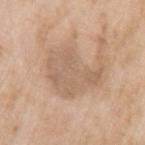workup: no biopsy performed (imaged during a skin exam)
automated lesion analysis: an average lesion color of about L≈61 a*≈16 b*≈30 (CIELAB), roughly 8 lightness units darker than nearby skin, and a normalized lesion–skin contrast near 5; a border-irregularity index near 6/10, a within-lesion color-variation index near 2.5/10, and a peripheral color-asymmetry measure near 1; a lesion-detection confidence of about 100/100
subject: female, aged 73 to 77
site: the left upper arm
acquisition: ~15 mm tile from a whole-body skin photo
lesion diameter: ~6.5 mm (longest diameter)